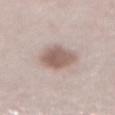notes — no biopsy performed (imaged during a skin exam)
image — ~15 mm crop, total-body skin-cancer survey
diameter — ≈4.5 mm
tile lighting — white-light
subject — female, in their 50s
body site — the abdomen
TBP lesion metrics — a border-irregularity index near 1.5/10, a within-lesion color-variation index near 3/10, and a peripheral color-asymmetry measure near 1; lesion-presence confidence of about 100/100